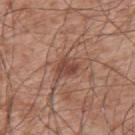The lesion was photographed on a routine skin check and not biopsied; there is no pathology result. A male patient, about 55 years old. A close-up tile cropped from a whole-body skin photograph, about 15 mm across. On the upper back.